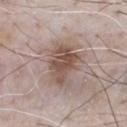The lesion was photographed on a routine skin check and not biopsied; there is no pathology result. A roughly 15 mm field-of-view crop from a total-body skin photograph. The patient is a male aged 53 to 57. Measured at roughly 5 mm in maximum diameter. Captured under white-light illumination. An algorithmic analysis of the crop reported a footprint of about 13 mm², an outline eccentricity of about 0.75 (0 = round, 1 = elongated), and a symmetry-axis asymmetry near 0.25. The analysis additionally found an average lesion color of about L≈51 a*≈16 b*≈23 (CIELAB) and a lesion-to-skin contrast of about 8.5 (normalized; higher = more distinct). The analysis additionally found a nevus-likeness score of about 65/100 and lesion-presence confidence of about 100/100.